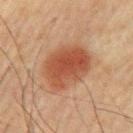Q: Was a biopsy performed?
A: catalogued during a skin exam; not biopsied
Q: Patient demographics?
A: male, aged 63–67
Q: Lesion location?
A: the left upper arm
Q: How was the tile lit?
A: cross-polarized
Q: How large is the lesion?
A: about 6 mm
Q: How was this image acquired?
A: ~15 mm crop, total-body skin-cancer survey